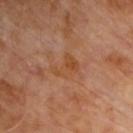Part of a total-body skin-imaging series; this lesion was reviewed on a skin check and was not flagged for biopsy. This is a cross-polarized tile. Cropped from a whole-body photographic skin survey; the tile spans about 15 mm. The patient is a male about 60 years old. From the upper back. Automated tile analysis of the lesion measured a mean CIELAB color near L≈45 a*≈22 b*≈35, about 5 CIELAB-L* units darker than the surrounding skin, and a normalized lesion–skin contrast near 6.5. The software also gave a within-lesion color-variation index near 2/10. The analysis additionally found a classifier nevus-likeness of about 0/100 and lesion-presence confidence of about 100/100.